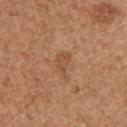The lesion was photographed on a routine skin check and not biopsied; there is no pathology result.
The tile uses white-light illumination.
The recorded lesion diameter is about 3 mm.
A roughly 15 mm field-of-view crop from a total-body skin photograph.
The lesion is located on the left upper arm.
The patient is a female aged approximately 40.